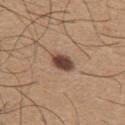Located on the chest. The lesion-visualizer software estimated a lesion color around L≈45 a*≈18 b*≈26 in CIELAB, roughly 16 lightness units darker than nearby skin, and a lesion-to-skin contrast of about 12 (normalized; higher = more distinct). It also reported a border-irregularity rating of about 2/10, internal color variation of about 4 on a 0–10 scale, and peripheral color asymmetry of about 1. A male patient, aged approximately 65. Cropped from a whole-body photographic skin survey; the tile spans about 15 mm. About 3 mm across.